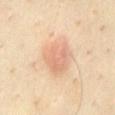The lesion was photographed on a routine skin check and not biopsied; there is no pathology result. A male patient approximately 40 years of age. The lesion is on the back. Automated image analysis of the tile measured border irregularity of about 2.5 on a 0–10 scale, a within-lesion color-variation index near 3.5/10, and peripheral color asymmetry of about 1. A close-up tile cropped from a whole-body skin photograph, about 15 mm across. Captured under cross-polarized illumination.